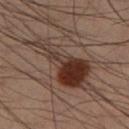follow-up: imaged on a skin check; not biopsied
diameter: ≈8.5 mm
subject: male, in their mid- to late 50s
imaging modality: ~15 mm crop, total-body skin-cancer survey
body site: the leg